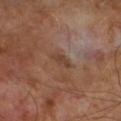workup: catalogued during a skin exam; not biopsied
automated metrics: an eccentricity of roughly 0.85
subject: male, approximately 65 years of age
diameter: about 3 mm
acquisition: ~15 mm crop, total-body skin-cancer survey
illumination: cross-polarized